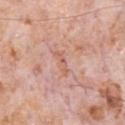{
  "biopsy_status": "not biopsied; imaged during a skin examination",
  "image": {
    "source": "total-body photography crop",
    "field_of_view_mm": 15
  },
  "patient": {
    "sex": "male",
    "age_approx": 80
  },
  "automated_metrics": {
    "area_mm2_approx": 2.0,
    "cielab_L": 60,
    "cielab_a": 25,
    "cielab_b": 29,
    "vs_skin_darker_L": 7.0,
    "vs_skin_contrast_norm": 5.0,
    "nevus_likeness_0_100": 0,
    "lesion_detection_confidence_0_100": 75
  },
  "lighting": "white-light",
  "site": "chest"
}